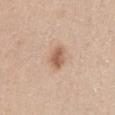biopsy status: imaged on a skin check; not biopsied
illumination: white-light illumination
image-analysis metrics: a lesion area of about 5 mm², an eccentricity of roughly 0.7, and a shape-asymmetry score of about 0.2 (0 = symmetric); a border-irregularity rating of about 1.5/10 and a color-variation rating of about 3/10; a classifier nevus-likeness of about 95/100
site: the chest
subject: female, in their mid- to late 40s
acquisition: ~15 mm tile from a whole-body skin photo
size: ≈3 mm